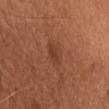Recorded during total-body skin imaging; not selected for excision or biopsy. The lesion is on the chest. About 3 mm across. The total-body-photography lesion software estimated an area of roughly 4 mm², an eccentricity of roughly 0.85, and two-axis asymmetry of about 0.2. Captured under white-light illumination. A male subject, in their mid-60s. A region of skin cropped from a whole-body photographic capture, roughly 15 mm wide.